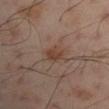notes = total-body-photography surveillance lesion; no biopsy
body site = the left arm
lighting = cross-polarized
patient = male, aged 48 to 52
lesion size = about 2.5 mm
image = 15 mm crop, total-body photography